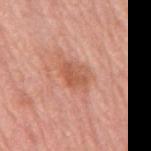follow-up = no biopsy performed (imaged during a skin exam)
anatomic site = the mid back
subject = female, about 75 years old
imaging modality = 15 mm crop, total-body photography